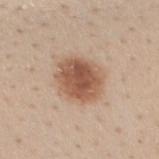Impression:
This lesion was catalogued during total-body skin photography and was not selected for biopsy.